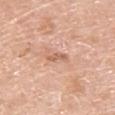Impression: Part of a total-body skin-imaging series; this lesion was reviewed on a skin check and was not flagged for biopsy. Clinical summary: From the upper back. A roughly 15 mm field-of-view crop from a total-body skin photograph. The subject is a male aged approximately 60.